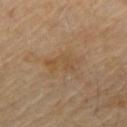Q: Was a biopsy performed?
A: no biopsy performed (imaged during a skin exam)
Q: What is the anatomic site?
A: the abdomen
Q: What kind of image is this?
A: 15 mm crop, total-body photography
Q: What are the patient's age and sex?
A: male, aged 58 to 62
Q: What is the lesion's diameter?
A: about 4 mm
Q: Illumination type?
A: cross-polarized illumination